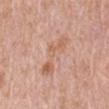Captured during whole-body skin photography for melanoma surveillance; the lesion was not biopsied. Captured under white-light illumination. Approximately 6.5 mm at its widest. Automated tile analysis of the lesion measured an area of roughly 12 mm², an outline eccentricity of about 0.95 (0 = round, 1 = elongated), and two-axis asymmetry of about 0.45. And it measured a border-irregularity index near 6/10, a color-variation rating of about 5/10, and a peripheral color-asymmetry measure near 1.5. And it measured a classifier nevus-likeness of about 0/100 and a detector confidence of about 100 out of 100 that the crop contains a lesion. A male subject aged 68–72. From the right upper arm. A 15 mm close-up tile from a total-body photography series done for melanoma screening.